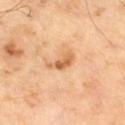{"biopsy_status": "not biopsied; imaged during a skin examination", "image": {"source": "total-body photography crop", "field_of_view_mm": 15}, "lighting": "cross-polarized", "patient": {"sex": "male", "age_approx": 65}, "lesion_size": {"long_diameter_mm_approx": 3.5}, "automated_metrics": {"area_mm2_approx": 4.0, "eccentricity": 0.9, "shape_asymmetry": 0.45, "vs_skin_darker_L": 12.0}}